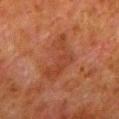The tile uses cross-polarized illumination. Located on the left lower leg. Measured at roughly 6 mm in maximum diameter. Automated tile analysis of the lesion measured a border-irregularity index near 6.5/10, a color-variation rating of about 2.5/10, and a peripheral color-asymmetry measure near 1. The software also gave a nevus-likeness score of about 0/100 and lesion-presence confidence of about 100/100. A lesion tile, about 15 mm wide, cut from a 3D total-body photograph. A male subject aged 78 to 82.